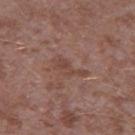biopsy status = catalogued during a skin exam; not biopsied
patient = male, in their mid- to late 40s
imaging modality = 15 mm crop, total-body photography
site = the left thigh
illumination = white-light
automated metrics = a symmetry-axis asymmetry near 0.45; border irregularity of about 6 on a 0–10 scale, a color-variation rating of about 2/10, and radial color variation of about 0.5